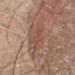Notes:
– notes · imaged on a skin check; not biopsied
– patient · male, aged 68–72
– image source · ~15 mm tile from a whole-body skin photo